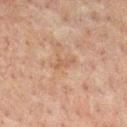Imaged during a routine full-body skin examination; the lesion was not biopsied and no histopathology is available. On the chest. Cropped from a total-body skin-imaging series; the visible field is about 15 mm. A male subject, aged approximately 60. Captured under cross-polarized illumination. Automated image analysis of the tile measured a shape eccentricity near 0.9. The analysis additionally found about 5 CIELAB-L* units darker than the surrounding skin. It also reported an automated nevus-likeness rating near 0 out of 100.